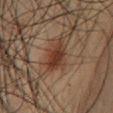This is a cross-polarized tile. Automated image analysis of the tile measured a classifier nevus-likeness of about 85/100. The lesion is located on the chest. A lesion tile, about 15 mm wide, cut from a 3D total-body photograph. A male patient approximately 60 years of age.